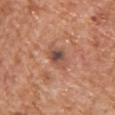Q: Was a biopsy performed?
A: catalogued during a skin exam; not biopsied
Q: Automated lesion metrics?
A: an average lesion color of about L≈50 a*≈22 b*≈28 (CIELAB) and a normalized lesion–skin contrast near 8; a color-variation rating of about 8/10 and peripheral color asymmetry of about 2
Q: How was this image acquired?
A: ~15 mm crop, total-body skin-cancer survey
Q: Illumination type?
A: white-light
Q: Where on the body is the lesion?
A: the front of the torso
Q: What are the patient's age and sex?
A: male, about 65 years old
Q: What is the lesion's diameter?
A: ~4 mm (longest diameter)